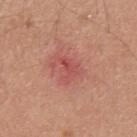Part of a total-body skin-imaging series; this lesion was reviewed on a skin check and was not flagged for biopsy.
From the upper back.
This image is a 15 mm lesion crop taken from a total-body photograph.
A male subject, aged 28–32.
Imaged with white-light lighting.
About 3 mm across.
Automated tile analysis of the lesion measured a border-irregularity index near 6/10, a within-lesion color-variation index near 3/10, and peripheral color asymmetry of about 1.5. The software also gave a nevus-likeness score of about 0/100.